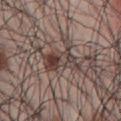patient = male, roughly 70 years of age; TBP lesion metrics = a nevus-likeness score of about 95/100; location = the front of the torso; lesion size = ≈5.5 mm; tile lighting = white-light illumination; acquisition = ~15 mm tile from a whole-body skin photo.